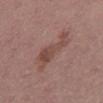No biopsy was performed on this lesion — it was imaged during a full skin examination and was not determined to be concerning. Imaged with white-light lighting. The patient is a male about 40 years old. The lesion-visualizer software estimated a lesion color around L≈47 a*≈20 b*≈23 in CIELAB, roughly 8 lightness units darker than nearby skin, and a normalized border contrast of about 6.5. The analysis additionally found a classifier nevus-likeness of about 5/100 and a detector confidence of about 100 out of 100 that the crop contains a lesion. On the abdomen. Measured at roughly 5.5 mm in maximum diameter. A region of skin cropped from a whole-body photographic capture, roughly 15 mm wide.